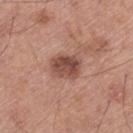Impression:
Part of a total-body skin-imaging series; this lesion was reviewed on a skin check and was not flagged for biopsy.
Image and clinical context:
The patient is a male roughly 65 years of age. Located on the leg. Cropped from a whole-body photographic skin survey; the tile spans about 15 mm.